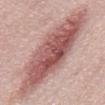  biopsy_status: not biopsied; imaged during a skin examination
  image:
    source: total-body photography crop
    field_of_view_mm: 15
  lighting: white-light
  patient:
    sex: male
    age_approx: 55
  automated_metrics:
    area_mm2_approx: 48.0
    eccentricity: 0.95
    shape_asymmetry: 0.15
    cielab_L: 56
    cielab_a: 24
    cielab_b: 23
    vs_skin_darker_L: 15.0
    vs_skin_contrast_norm: 9.5
  site: abdomen